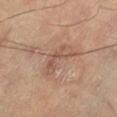biopsy status: catalogued during a skin exam; not biopsied
subject: male, aged 58 to 62
lesion diameter: ~5 mm (longest diameter)
anatomic site: the leg
lighting: cross-polarized
imaging modality: ~15 mm tile from a whole-body skin photo